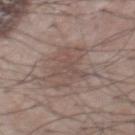Image and clinical context: The lesion is located on the front of the torso. A lesion tile, about 15 mm wide, cut from a 3D total-body photograph. A male subject in their mid-50s. Captured under white-light illumination.